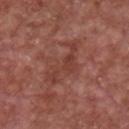• imaging modality · ~15 mm crop, total-body skin-cancer survey
• body site · the chest
• patient · male, aged around 65